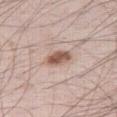Q: Is there a histopathology result?
A: total-body-photography surveillance lesion; no biopsy
Q: What did automated image analysis measure?
A: a footprint of about 5 mm², an eccentricity of roughly 0.8, and a symmetry-axis asymmetry near 0.2; a lesion color around L≈55 a*≈19 b*≈26 in CIELAB, a lesion–skin lightness drop of about 14, and a normalized lesion–skin contrast near 9.5; a border-irregularity index near 2/10 and peripheral color asymmetry of about 1
Q: What are the patient's age and sex?
A: male, roughly 60 years of age
Q: Where on the body is the lesion?
A: the right thigh
Q: What lighting was used for the tile?
A: white-light illumination
Q: How large is the lesion?
A: ~3 mm (longest diameter)
Q: What kind of image is this?
A: 15 mm crop, total-body photography